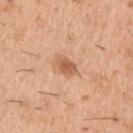notes — no biopsy performed (imaged during a skin exam)
subject — male, about 40 years old
body site — the left upper arm
automated lesion analysis — a mean CIELAB color near L≈59 a*≈24 b*≈35, about 12 CIELAB-L* units darker than the surrounding skin, and a lesion-to-skin contrast of about 7.5 (normalized; higher = more distinct); a border-irregularity index near 1.5/10, a color-variation rating of about 3/10, and a peripheral color-asymmetry measure near 1; a nevus-likeness score of about 75/100
image — ~15 mm tile from a whole-body skin photo
tile lighting — white-light illumination